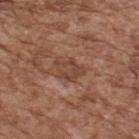imaging modality: 15 mm crop, total-body photography
site: the upper back
size: ~3.5 mm (longest diameter)
subject: male, in their mid-70s
illumination: white-light illumination